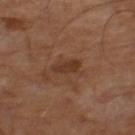| key | value |
|---|---|
| follow-up | no biopsy performed (imaged during a skin exam) |
| patient | male, in their mid- to late 60s |
| tile lighting | cross-polarized |
| acquisition | ~15 mm tile from a whole-body skin photo |
| image-analysis metrics | a lesion color around L≈33 a*≈20 b*≈28 in CIELAB, a lesion–skin lightness drop of about 8, and a normalized border contrast of about 7; a classifier nevus-likeness of about 0/100 and lesion-presence confidence of about 100/100 |
| diameter | about 3 mm |